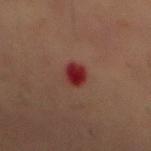follow-up: no biopsy performed (imaged during a skin exam)
location: the abdomen
illumination: cross-polarized illumination
lesion size: about 3 mm
subject: male, in their mid- to late 50s
automated metrics: a footprint of about 5 mm², an outline eccentricity of about 0.7 (0 = round, 1 = elongated), and two-axis asymmetry of about 0.2; a lesion color around L≈26 a*≈28 b*≈21 in CIELAB, a lesion–skin lightness drop of about 11, and a normalized lesion–skin contrast near 11.5; a border-irregularity rating of about 1.5/10
imaging modality: total-body-photography crop, ~15 mm field of view